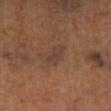Captured during whole-body skin photography for melanoma surveillance; the lesion was not biopsied. The lesion is located on the left lower leg. The tile uses cross-polarized illumination. A female patient, aged approximately 65. A lesion tile, about 15 mm wide, cut from a 3D total-body photograph. The lesion's longest dimension is about 3 mm.